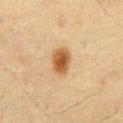The lesion is on the abdomen. Imaged with cross-polarized lighting. The patient is a male aged 58–62. A 15 mm crop from a total-body photograph taken for skin-cancer surveillance.